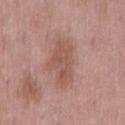Recorded during total-body skin imaging; not selected for excision or biopsy. The patient is a male in their mid-50s. Automated tile analysis of the lesion measured border irregularity of about 3 on a 0–10 scale, a within-lesion color-variation index near 3/10, and peripheral color asymmetry of about 1. The lesion is on the back. A 15 mm close-up tile from a total-body photography series done for melanoma screening. Measured at roughly 5.5 mm in maximum diameter. Imaged with white-light lighting.